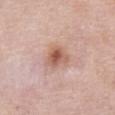Captured during whole-body skin photography for melanoma surveillance; the lesion was not biopsied.
About 3.5 mm across.
A 15 mm close-up tile from a total-body photography series done for melanoma screening.
Automated image analysis of the tile measured an average lesion color of about L≈58 a*≈22 b*≈29 (CIELAB), about 12 CIELAB-L* units darker than the surrounding skin, and a normalized lesion–skin contrast near 8. The analysis additionally found internal color variation of about 6 on a 0–10 scale. The software also gave an automated nevus-likeness rating near 85 out of 100 and a lesion-detection confidence of about 100/100.
The tile uses white-light illumination.
The lesion is on the abdomen.
The patient is a female aged 58 to 62.